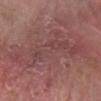Captured during whole-body skin photography for melanoma surveillance; the lesion was not biopsied.
The patient is a female aged 83 or over.
From the right forearm.
A roughly 15 mm field-of-view crop from a total-body skin photograph.